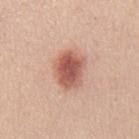biopsy_status: not biopsied; imaged during a skin examination